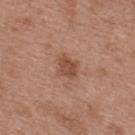Captured during whole-body skin photography for melanoma surveillance; the lesion was not biopsied.
Measured at roughly 2.5 mm in maximum diameter.
Imaged with white-light lighting.
Cropped from a total-body skin-imaging series; the visible field is about 15 mm.
A female patient roughly 40 years of age.
Automated tile analysis of the lesion measured a lesion area of about 5 mm², a shape eccentricity near 0.6, and two-axis asymmetry of about 0.3. It also reported a lesion–skin lightness drop of about 10 and a normalized lesion–skin contrast near 7.5. The software also gave border irregularity of about 3 on a 0–10 scale, a within-lesion color-variation index near 2/10, and radial color variation of about 0.5.
The lesion is on the upper back.